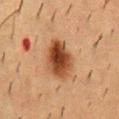Impression: Captured during whole-body skin photography for melanoma surveillance; the lesion was not biopsied. Acquisition and patient details: On the back. A 15 mm close-up extracted from a 3D total-body photography capture. Approximately 5 mm at its widest. Automated tile analysis of the lesion measured a border-irregularity index near 2/10 and a color-variation rating of about 8/10. The analysis additionally found a detector confidence of about 100 out of 100 that the crop contains a lesion. Imaged with cross-polarized lighting. The subject is a male aged approximately 55.